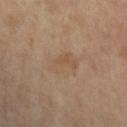The total-body-photography lesion software estimated a footprint of about 6 mm² and an outline eccentricity of about 0.85 (0 = round, 1 = elongated). And it measured a normalized border contrast of about 5. It also reported a border-irregularity index near 4/10, internal color variation of about 2 on a 0–10 scale, and radial color variation of about 0.5. It also reported a classifier nevus-likeness of about 0/100. Longest diameter approximately 4 mm. The lesion is on the left lower leg. The patient is a female about 75 years old. A lesion tile, about 15 mm wide, cut from a 3D total-body photograph. The tile uses cross-polarized illumination.